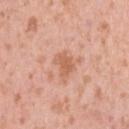Clinical impression: Recorded during total-body skin imaging; not selected for excision or biopsy. Image and clinical context: About 3 mm across. This is a white-light tile. From the left upper arm. Automated image analysis of the tile measured a mean CIELAB color near L≈61 a*≈25 b*≈34, about 9 CIELAB-L* units darker than the surrounding skin, and a normalized lesion–skin contrast near 6.5. The software also gave an automated nevus-likeness rating near 5 out of 100 and a lesion-detection confidence of about 100/100. Cropped from a whole-body photographic skin survey; the tile spans about 15 mm. A female subject, in their 40s.